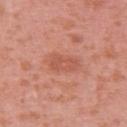This lesion was catalogued during total-body skin photography and was not selected for biopsy.
A region of skin cropped from a whole-body photographic capture, roughly 15 mm wide.
Located on the upper back.
The tile uses white-light illumination.
Measured at roughly 4.5 mm in maximum diameter.
A female patient, approximately 40 years of age.